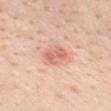follow-up: no biopsy performed (imaged during a skin exam)
patient: female, about 45 years old
image: 15 mm crop, total-body photography
lighting: white-light
automated metrics: an area of roughly 6 mm², an outline eccentricity of about 0.8 (0 = round, 1 = elongated), and a symmetry-axis asymmetry near 0.2; a lesion color around L≈66 a*≈26 b*≈31 in CIELAB, roughly 10 lightness units darker than nearby skin, and a normalized lesion–skin contrast near 6; a classifier nevus-likeness of about 85/100 and a lesion-detection confidence of about 100/100
body site: the chest
lesion diameter: ~3.5 mm (longest diameter)